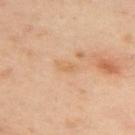<tbp_lesion>
  <biopsy_status>not biopsied; imaged during a skin examination</biopsy_status>
  <lighting>cross-polarized</lighting>
  <image>
    <source>total-body photography crop</source>
    <field_of_view_mm>15</field_of_view_mm>
  </image>
  <automated_metrics>
    <area_mm2_approx>2.5</area_mm2_approx>
    <nevus_likeness_0_100>0</nevus_likeness_0_100>
    <lesion_detection_confidence_0_100>100</lesion_detection_confidence_0_100>
  </automated_metrics>
  <site>upper back</site>
  <patient>
    <sex>female</sex>
    <age_approx>40</age_approx>
  </patient>
  <lesion_size>
    <long_diameter_mm_approx>2.5</long_diameter_mm_approx>
  </lesion_size>
</tbp_lesion>